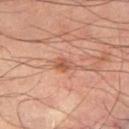{
  "biopsy_status": "not biopsied; imaged during a skin examination",
  "image": {
    "source": "total-body photography crop",
    "field_of_view_mm": 15
  },
  "lesion_size": {
    "long_diameter_mm_approx": 2.5
  },
  "site": "right thigh",
  "lighting": "cross-polarized",
  "patient": {
    "sex": "male",
    "age_approx": 65
  }
}